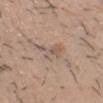The lesion was tiled from a total-body skin photograph and was not biopsied. The total-body-photography lesion software estimated an average lesion color of about L≈56 a*≈15 b*≈26 (CIELAB), a lesion–skin lightness drop of about 7, and a normalized lesion–skin contrast near 5. And it measured a border-irregularity rating of about 7.5/10, a within-lesion color-variation index near 4.5/10, and peripheral color asymmetry of about 1.5. The patient is a male in their mid- to late 30s. On the head or neck. The tile uses white-light illumination. A close-up tile cropped from a whole-body skin photograph, about 15 mm across.